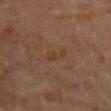– workup: catalogued during a skin exam; not biopsied
– subject: female, roughly 80 years of age
– tile lighting: cross-polarized
– lesion size: ~3 mm (longest diameter)
– acquisition: ~15 mm tile from a whole-body skin photo
– site: the chest